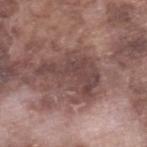The lesion was photographed on a routine skin check and not biopsied; there is no pathology result. Automated image analysis of the tile measured a footprint of about 19 mm², a shape eccentricity near 0.8, and two-axis asymmetry of about 0.45. And it measured a within-lesion color-variation index near 3/10 and a peripheral color-asymmetry measure near 1. A roughly 15 mm field-of-view crop from a total-body skin photograph. The lesion is on the right lower leg. A male patient in their mid-70s. Measured at roughly 6.5 mm in maximum diameter. Captured under white-light illumination.